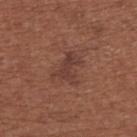Captured during whole-body skin photography for melanoma surveillance; the lesion was not biopsied. A female subject, in their mid- to late 60s. Located on the upper back. A 15 mm close-up tile from a total-body photography series done for melanoma screening. Captured under white-light illumination.